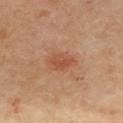No biopsy was performed on this lesion — it was imaged during a full skin examination and was not determined to be concerning. A lesion tile, about 15 mm wide, cut from a 3D total-body photograph. The lesion is on the front of the torso. Measured at roughly 3.5 mm in maximum diameter. The subject is a female in their 40s.